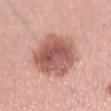Impression:
No biopsy was performed on this lesion — it was imaged during a full skin examination and was not determined to be concerning.
Clinical summary:
A female patient, in their mid-40s. This is a white-light tile. The lesion-visualizer software estimated a footprint of about 26 mm² and a shape-asymmetry score of about 0.15 (0 = symmetric). The analysis additionally found an average lesion color of about L≈57 a*≈25 b*≈25 (CIELAB) and about 15 CIELAB-L* units darker than the surrounding skin. The software also gave a classifier nevus-likeness of about 30/100 and lesion-presence confidence of about 100/100. Cropped from a whole-body photographic skin survey; the tile spans about 15 mm. Located on the right thigh.